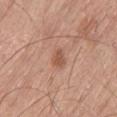The lesion was tiled from a total-body skin photograph and was not biopsied.
From the left thigh.
Captured under white-light illumination.
The lesion's longest dimension is about 3 mm.
A male subject approximately 80 years of age.
A 15 mm close-up extracted from a 3D total-body photography capture.